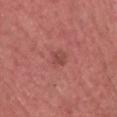The lesion was tiled from a total-body skin photograph and was not biopsied.
The lesion is located on the head or neck.
Longest diameter approximately 2.5 mm.
A male patient, about 70 years old.
An algorithmic analysis of the crop reported lesion-presence confidence of about 100/100.
Imaged with white-light lighting.
A lesion tile, about 15 mm wide, cut from a 3D total-body photograph.